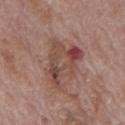Part of a total-body skin-imaging series; this lesion was reviewed on a skin check and was not flagged for biopsy. Approximately 6 mm at its widest. On the back. A 15 mm close-up tile from a total-body photography series done for melanoma screening. A male patient aged 68–72. Captured under white-light illumination.